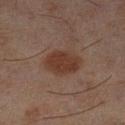Clinical impression: Imaged during a routine full-body skin examination; the lesion was not biopsied and no histopathology is available. Clinical summary: Located on the left lower leg. A 15 mm close-up extracted from a 3D total-body photography capture. About 4 mm across. The lesion-visualizer software estimated a nevus-likeness score of about 95/100 and a lesion-detection confidence of about 100/100. Captured under cross-polarized illumination. A male subject aged 43–47.